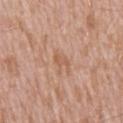Notes:
* workup — no biopsy performed (imaged during a skin exam)
* body site — the abdomen
* patient — male, roughly 60 years of age
* lesion size — ≈3 mm
* lighting — white-light
* image source — 15 mm crop, total-body photography